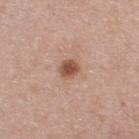Q: Where on the body is the lesion?
A: the upper back
Q: What are the patient's age and sex?
A: male, aged 43 to 47
Q: What lighting was used for the tile?
A: white-light
Q: What kind of image is this?
A: ~15 mm crop, total-body skin-cancer survey